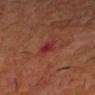Part of a total-body skin-imaging series; this lesion was reviewed on a skin check and was not flagged for biopsy.
On the right forearm.
Cropped from a total-body skin-imaging series; the visible field is about 15 mm.
This is a cross-polarized tile.
Automated image analysis of the tile measured an average lesion color of about L≈31 a*≈30 b*≈25 (CIELAB), a lesion–skin lightness drop of about 7, and a lesion-to-skin contrast of about 7 (normalized; higher = more distinct). And it measured border irregularity of about 2.5 on a 0–10 scale, a within-lesion color-variation index near 3.5/10, and radial color variation of about 1.5.
A male subject, aged 58 to 62.